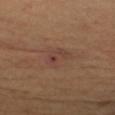Clinical impression:
No biopsy was performed on this lesion — it was imaged during a full skin examination and was not determined to be concerning.
Clinical summary:
Imaged with cross-polarized lighting. The patient is a female about 40 years old. Cropped from a whole-body photographic skin survey; the tile spans about 15 mm. Approximately 3.5 mm at its widest. On the left upper arm.